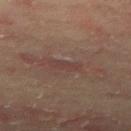Background: The lesion is located on the right thigh. The subject is a male roughly 65 years of age. A 15 mm close-up tile from a total-body photography series done for melanoma screening.